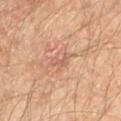The lesion was photographed on a routine skin check and not biopsied; there is no pathology result.
A male patient in their mid-60s.
Longest diameter approximately 2.5 mm.
This image is a 15 mm lesion crop taken from a total-body photograph.
This is a cross-polarized tile.
Located on the arm.